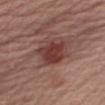Clinical impression: The lesion was tiled from a total-body skin photograph and was not biopsied. Clinical summary: This is a white-light tile. An algorithmic analysis of the crop reported a lesion–skin lightness drop of about 11. On the right upper arm. Longest diameter approximately 4.5 mm. A male patient, approximately 60 years of age. Cropped from a whole-body photographic skin survey; the tile spans about 15 mm.